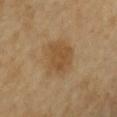Impression: This lesion was catalogued during total-body skin photography and was not selected for biopsy. Background: A close-up tile cropped from a whole-body skin photograph, about 15 mm across. The tile uses cross-polarized illumination. From the chest. A female patient, about 70 years old. Approximately 4.5 mm at its widest.